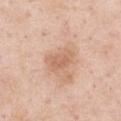Impression:
Recorded during total-body skin imaging; not selected for excision or biopsy.
Clinical summary:
A male patient aged around 55. Approximately 3.5 mm at its widest. Automated tile analysis of the lesion measured a mean CIELAB color near L≈64 a*≈21 b*≈32, roughly 9 lightness units darker than nearby skin, and a lesion-to-skin contrast of about 6 (normalized; higher = more distinct). The analysis additionally found a border-irregularity index near 3.5/10, a within-lesion color-variation index near 2/10, and a peripheral color-asymmetry measure near 0.5. The lesion is located on the right upper arm. A lesion tile, about 15 mm wide, cut from a 3D total-body photograph. The tile uses white-light illumination.